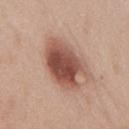follow-up: total-body-photography surveillance lesion; no biopsy | subject: female, in their 40s | imaging modality: ~15 mm tile from a whole-body skin photo | image-analysis metrics: an average lesion color of about L≈51 a*≈22 b*≈27 (CIELAB) and a normalized lesion–skin contrast near 10.5; border irregularity of about 3.5 on a 0–10 scale; an automated nevus-likeness rating near 95 out of 100 and a detector confidence of about 100 out of 100 that the crop contains a lesion | location: the chest | lesion size: ≈7 mm.